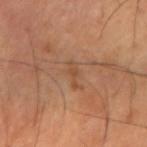The lesion was photographed on a routine skin check and not biopsied; there is no pathology result. A male patient, aged approximately 60. The lesion's longest dimension is about 3 mm. The lesion is located on the right forearm. The total-body-photography lesion software estimated about 6 CIELAB-L* units darker than the surrounding skin and a normalized lesion–skin contrast near 5. The software also gave a classifier nevus-likeness of about 0/100. Imaged with cross-polarized lighting. A region of skin cropped from a whole-body photographic capture, roughly 15 mm wide.